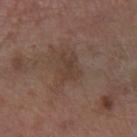{
  "biopsy_status": "not biopsied; imaged during a skin examination",
  "lighting": "white-light",
  "site": "left forearm",
  "image": {
    "source": "total-body photography crop",
    "field_of_view_mm": 15
  },
  "lesion_size": {
    "long_diameter_mm_approx": 3.0
  },
  "patient": {
    "sex": "male",
    "age_approx": 55
  }
}